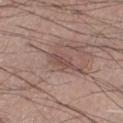No biopsy was performed on this lesion — it was imaged during a full skin examination and was not determined to be concerning.
Automated image analysis of the tile measured an area of roughly 4 mm², a shape eccentricity near 0.95, and a symmetry-axis asymmetry near 0.3. The analysis additionally found a lesion color around L≈48 a*≈18 b*≈22 in CIELAB and a normalized border contrast of about 6. The software also gave a border-irregularity rating of about 4/10 and a peripheral color-asymmetry measure near 0. The software also gave a nevus-likeness score of about 0/100 and a detector confidence of about 95 out of 100 that the crop contains a lesion.
The tile uses white-light illumination.
A lesion tile, about 15 mm wide, cut from a 3D total-body photograph.
A male subject, approximately 55 years of age.